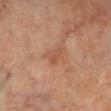Q: Was a biopsy performed?
A: total-body-photography surveillance lesion; no biopsy
Q: Patient demographics?
A: male, about 70 years old
Q: Lesion location?
A: the right lower leg
Q: What is the imaging modality?
A: total-body-photography crop, ~15 mm field of view